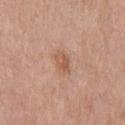This lesion was catalogued during total-body skin photography and was not selected for biopsy. From the chest. The lesion-visualizer software estimated border irregularity of about 2.5 on a 0–10 scale, internal color variation of about 4 on a 0–10 scale, and radial color variation of about 1.5. The analysis additionally found an automated nevus-likeness rating near 10 out of 100 and a detector confidence of about 100 out of 100 that the crop contains a lesion. The lesion's longest dimension is about 3 mm. A 15 mm close-up extracted from a 3D total-body photography capture. A male subject, in their mid-50s.